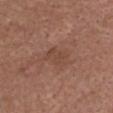<tbp_lesion>
<biopsy_status>not biopsied; imaged during a skin examination</biopsy_status>
<site>head or neck</site>
<image>
  <source>total-body photography crop</source>
  <field_of_view_mm>15</field_of_view_mm>
</image>
<patient>
  <sex>male</sex>
  <age_approx>75</age_approx>
</patient>
<lesion_size>
  <long_diameter_mm_approx>3.0</long_diameter_mm_approx>
</lesion_size>
</tbp_lesion>